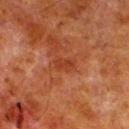follow-up: no biopsy performed (imaged during a skin exam); patient: male, in their 80s; location: the left lower leg; tile lighting: cross-polarized illumination; imaging modality: ~15 mm tile from a whole-body skin photo.